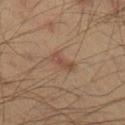follow-up: no biopsy performed (imaged during a skin exam)
illumination: cross-polarized
automated lesion analysis: an outline eccentricity of about 0.9 (0 = round, 1 = elongated) and two-axis asymmetry of about 0.3; a lesion color around L≈48 a*≈19 b*≈28 in CIELAB, a lesion–skin lightness drop of about 8, and a lesion-to-skin contrast of about 6 (normalized; higher = more distinct); internal color variation of about 2 on a 0–10 scale and peripheral color asymmetry of about 0.5; a lesion-detection confidence of about 100/100
diameter: ~3 mm (longest diameter)
location: the right lower leg
acquisition: ~15 mm tile from a whole-body skin photo
subject: male, about 40 years old